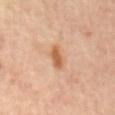Q: Was a biopsy performed?
A: total-body-photography surveillance lesion; no biopsy
Q: Automated lesion metrics?
A: a classifier nevus-likeness of about 70/100
Q: What lighting was used for the tile?
A: cross-polarized illumination
Q: What is the lesion's diameter?
A: about 3.5 mm
Q: Where on the body is the lesion?
A: the mid back
Q: Patient demographics?
A: male, aged around 60
Q: What kind of image is this?
A: ~15 mm tile from a whole-body skin photo